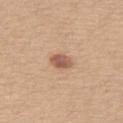Imaged during a routine full-body skin examination; the lesion was not biopsied and no histopathology is available.
The lesion is located on the left thigh.
The recorded lesion diameter is about 3 mm.
Cropped from a whole-body photographic skin survey; the tile spans about 15 mm.
The total-body-photography lesion software estimated roughly 13 lightness units darker than nearby skin. It also reported a border-irregularity index near 1.5/10, a within-lesion color-variation index near 3/10, and peripheral color asymmetry of about 1.
Imaged with white-light lighting.
The subject is a female in their 60s.